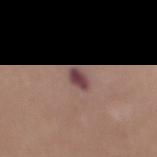Part of a total-body skin-imaging series; this lesion was reviewed on a skin check and was not flagged for biopsy. Approximately 2.5 mm at its widest. This image is a 15 mm lesion crop taken from a total-body photograph. Captured under white-light illumination. A male subject, roughly 30 years of age. Automated image analysis of the tile measured an average lesion color of about L≈44 a*≈21 b*≈17 (CIELAB), roughly 13 lightness units darker than nearby skin, and a normalized lesion–skin contrast near 11. The analysis additionally found a color-variation rating of about 2.5/10 and a peripheral color-asymmetry measure near 1. The analysis additionally found an automated nevus-likeness rating near 15 out of 100 and a lesion-detection confidence of about 100/100. The lesion is located on the mid back.